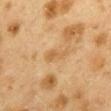Clinical impression:
Recorded during total-body skin imaging; not selected for excision or biopsy.
Image and clinical context:
About 3 mm across. A region of skin cropped from a whole-body photographic capture, roughly 15 mm wide. The patient is a female roughly 40 years of age. On the mid back.